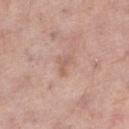  biopsy_status: not biopsied; imaged during a skin examination
  lighting: white-light
  automated_metrics:
    area_mm2_approx: 3.5
    eccentricity: 0.75
    shape_asymmetry: 0.4
    vs_skin_darker_L: 7.0
    vs_skin_contrast_norm: 5.0
    border_irregularity_0_10: 4.0
    color_variation_0_10: 1.5
    peripheral_color_asymmetry: 0.5
    lesion_detection_confidence_0_100: 100
  lesion_size:
    long_diameter_mm_approx: 2.5
  patient:
    sex: female
    age_approx: 60
  site: left thigh
  image:
    source: total-body photography crop
    field_of_view_mm: 15